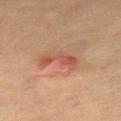Assessment:
The lesion was tiled from a total-body skin photograph and was not biopsied.
Context:
A male patient in their 70s. A 15 mm close-up extracted from a 3D total-body photography capture. The lesion is on the mid back.